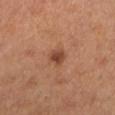  biopsy_status: not biopsied; imaged during a skin examination
  site: left lower leg
  patient:
    sex: male
    age_approx: 65
  image:
    source: total-body photography crop
    field_of_view_mm: 15
  lesion_size:
    long_diameter_mm_approx: 2.5
  automated_metrics:
    area_mm2_approx: 3.5
    eccentricity: 0.6
    shape_asymmetry: 0.15
    cielab_L: 42
    cielab_a: 23
    cielab_b: 30
    vs_skin_darker_L: 10.0
    border_irregularity_0_10: 1.0
    color_variation_0_10: 2.0
    peripheral_color_asymmetry: 0.5
    nevus_likeness_0_100: 85
    lesion_detection_confidence_0_100: 100
  lighting: cross-polarized